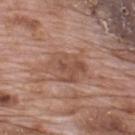Impression:
Part of a total-body skin-imaging series; this lesion was reviewed on a skin check and was not flagged for biopsy.
Background:
The subject is a male in their 70s. On the upper back. Approximately 6 mm at its widest. The lesion-visualizer software estimated a border-irregularity rating of about 3.5/10, internal color variation of about 4 on a 0–10 scale, and peripheral color asymmetry of about 1.5. The analysis additionally found lesion-presence confidence of about 85/100. A lesion tile, about 15 mm wide, cut from a 3D total-body photograph.